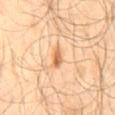Imaged during a routine full-body skin examination; the lesion was not biopsied and no histopathology is available. The patient is a male about 65 years old. This is a cross-polarized tile. The lesion is on the mid back. Measured at roughly 2.5 mm in maximum diameter. A close-up tile cropped from a whole-body skin photograph, about 15 mm across.